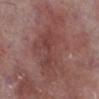automated metrics: a lesion color around L≈43 a*≈23 b*≈22 in CIELAB and a normalized lesion–skin contrast near 6; a border-irregularity index near 7/10, a color-variation rating of about 3.5/10, and a peripheral color-asymmetry measure near 1 | site: the right lower leg | acquisition: ~15 mm crop, total-body skin-cancer survey | lesion diameter: about 9.5 mm | patient: male, roughly 75 years of age.